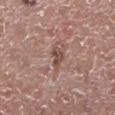- workup — no biopsy performed (imaged during a skin exam)
- lesion size — ≈2.5 mm
- imaging modality — ~15 mm crop, total-body skin-cancer survey
- site — the right lower leg
- tile lighting — white-light
- patient — male, aged 53 to 57